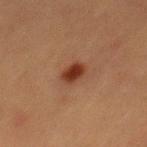Clinical impression: Part of a total-body skin-imaging series; this lesion was reviewed on a skin check and was not flagged for biopsy. Acquisition and patient details: The lesion is on the back. Automated image analysis of the tile measured a border-irregularity index near 1.5/10, internal color variation of about 2 on a 0–10 scale, and radial color variation of about 0.5. The software also gave an automated nevus-likeness rating near 100 out of 100 and lesion-presence confidence of about 100/100. Cropped from a total-body skin-imaging series; the visible field is about 15 mm. A male subject roughly 40 years of age. Longest diameter approximately 2.5 mm.